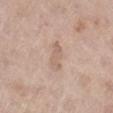Assessment: The lesion was tiled from a total-body skin photograph and was not biopsied.